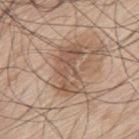biopsy status: total-body-photography surveillance lesion; no biopsy
automated metrics: an eccentricity of roughly 0.85 and a shape-asymmetry score of about 0.4 (0 = symmetric); a border-irregularity index near 5.5/10, a color-variation rating of about 5.5/10, and radial color variation of about 2; a detector confidence of about 90 out of 100 that the crop contains a lesion
patient: male, roughly 70 years of age
anatomic site: the mid back
lesion diameter: ~6 mm (longest diameter)
lighting: white-light illumination
acquisition: ~15 mm crop, total-body skin-cancer survey